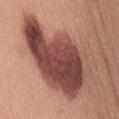The lesion was photographed on a routine skin check and not biopsied; there is no pathology result.
Cropped from a whole-body photographic skin survey; the tile spans about 15 mm.
Approximately 12 mm at its widest.
Captured under white-light illumination.
A female patient, aged approximately 40.
Located on the mid back.
Automated image analysis of the tile measured a lesion area of about 55 mm², a shape eccentricity near 0.85, and a symmetry-axis asymmetry near 0.35. The analysis additionally found a mean CIELAB color near L≈46 a*≈24 b*≈25 and a normalized lesion–skin contrast near 12. The analysis additionally found internal color variation of about 9 on a 0–10 scale and a peripheral color-asymmetry measure near 3.5. The analysis additionally found a nevus-likeness score of about 30/100 and lesion-presence confidence of about 100/100.